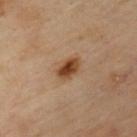Image and clinical context: Located on the chest. The subject is a male aged approximately 55. Cropped from a whole-body photographic skin survey; the tile spans about 15 mm.